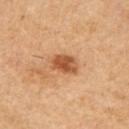<tbp_lesion>
  <patient>
    <sex>male</sex>
    <age_approx>55</age_approx>
  </patient>
  <site>right upper arm</site>
  <automated_metrics>
    <area_mm2_approx>6.5</area_mm2_approx>
    <eccentricity>0.6</eccentricity>
    <shape_asymmetry>0.25</shape_asymmetry>
    <nevus_likeness_0_100>85</nevus_likeness_0_100>
    <lesion_detection_confidence_0_100>100</lesion_detection_confidence_0_100>
  </automated_metrics>
  <lesion_size>
    <long_diameter_mm_approx>3.0</long_diameter_mm_approx>
  </lesion_size>
  <image>
    <source>total-body photography crop</source>
    <field_of_view_mm>15</field_of_view_mm>
  </image>
</tbp_lesion>